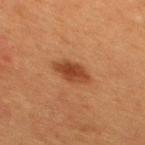Impression:
Recorded during total-body skin imaging; not selected for excision or biopsy.
Acquisition and patient details:
On the upper back. The total-body-photography lesion software estimated a lesion area of about 7.5 mm² and two-axis asymmetry of about 0.15. It also reported about 10 CIELAB-L* units darker than the surrounding skin and a lesion-to-skin contrast of about 8.5 (normalized; higher = more distinct). And it measured a within-lesion color-variation index near 3/10 and radial color variation of about 1. A female patient, roughly 40 years of age. Imaged with cross-polarized lighting. A 15 mm crop from a total-body photograph taken for skin-cancer surveillance.